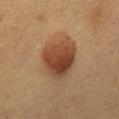Clinical impression: Captured during whole-body skin photography for melanoma surveillance; the lesion was not biopsied. Image and clinical context: On the mid back. A 15 mm close-up extracted from a 3D total-body photography capture. The total-body-photography lesion software estimated a lesion color around L≈35 a*≈18 b*≈27 in CIELAB and about 11 CIELAB-L* units darker than the surrounding skin. The analysis additionally found border irregularity of about 2 on a 0–10 scale, a color-variation rating of about 6/10, and a peripheral color-asymmetry measure near 2. And it measured a nevus-likeness score of about 100/100 and lesion-presence confidence of about 100/100. A female patient, aged approximately 50.